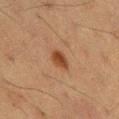Assessment:
Part of a total-body skin-imaging series; this lesion was reviewed on a skin check and was not flagged for biopsy.
Background:
An algorithmic analysis of the crop reported an average lesion color of about L≈35 a*≈19 b*≈28 (CIELAB), roughly 9 lightness units darker than nearby skin, and a normalized border contrast of about 9. The analysis additionally found border irregularity of about 1.5 on a 0–10 scale and peripheral color asymmetry of about 1. A close-up tile cropped from a whole-body skin photograph, about 15 mm across. The tile uses cross-polarized illumination. A male subject aged around 60. The recorded lesion diameter is about 3 mm. Located on the back.